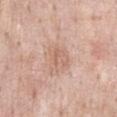{"biopsy_status": "not biopsied; imaged during a skin examination", "lesion_size": {"long_diameter_mm_approx": 3.5}, "image": {"source": "total-body photography crop", "field_of_view_mm": 15}, "lighting": "white-light", "site": "chest", "patient": {"sex": "male", "age_approx": 55}}